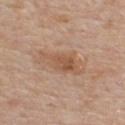{"biopsy_status": "not biopsied; imaged during a skin examination", "patient": {"sex": "male", "age_approx": 60}, "image": {"source": "total-body photography crop", "field_of_view_mm": 15}, "site": "chest"}